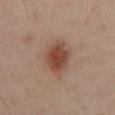Part of a total-body skin-imaging series; this lesion was reviewed on a skin check and was not flagged for biopsy. A male subject, in their mid-50s. The lesion-visualizer software estimated an outline eccentricity of about 0.7 (0 = round, 1 = elongated). The analysis additionally found a mean CIELAB color near L≈45 a*≈22 b*≈28 and roughly 12 lightness units darker than nearby skin. And it measured a border-irregularity rating of about 2/10, internal color variation of about 3 on a 0–10 scale, and a peripheral color-asymmetry measure near 1. The software also gave lesion-presence confidence of about 100/100. Longest diameter approximately 4.5 mm. A lesion tile, about 15 mm wide, cut from a 3D total-body photograph. The lesion is on the back. Captured under cross-polarized illumination.